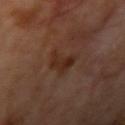Imaged during a routine full-body skin examination; the lesion was not biopsied and no histopathology is available. The lesion's longest dimension is about 4 mm. The lesion is on the right upper arm. A 15 mm crop from a total-body photograph taken for skin-cancer surveillance. An algorithmic analysis of the crop reported a lesion area of about 6.5 mm², a shape eccentricity near 0.8, and two-axis asymmetry of about 0.4. The software also gave a border-irregularity rating of about 4.5/10 and internal color variation of about 3.5 on a 0–10 scale. Captured under cross-polarized illumination. A male subject approximately 70 years of age.